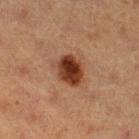Recorded during total-body skin imaging; not selected for excision or biopsy. This image is a 15 mm lesion crop taken from a total-body photograph. Automated image analysis of the tile measured an eccentricity of roughly 0.55. The software also gave a mean CIELAB color near L≈32 a*≈20 b*≈27, about 13 CIELAB-L* units darker than the surrounding skin, and a lesion-to-skin contrast of about 11.5 (normalized; higher = more distinct). The analysis additionally found border irregularity of about 1.5 on a 0–10 scale, a color-variation rating of about 6.5/10, and a peripheral color-asymmetry measure near 2. The tile uses cross-polarized illumination. From the right thigh. A female subject, aged 38 to 42. The recorded lesion diameter is about 4 mm.